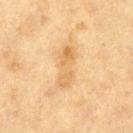notes: no biopsy performed (imaged during a skin exam)
automated metrics: a lesion area of about 8 mm², a shape eccentricity near 0.95, and a symmetry-axis asymmetry near 0.3; border irregularity of about 4 on a 0–10 scale, internal color variation of about 4 on a 0–10 scale, and radial color variation of about 1.5
imaging modality: 15 mm crop, total-body photography
site: the left thigh
patient: female, aged 38–42